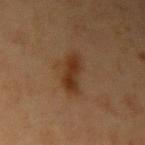Recorded during total-body skin imaging; not selected for excision or biopsy.
A female patient about 40 years old.
Located on the left upper arm.
Captured under cross-polarized illumination.
A region of skin cropped from a whole-body photographic capture, roughly 15 mm wide.